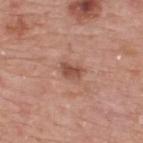No biopsy was performed on this lesion — it was imaged during a full skin examination and was not determined to be concerning. The lesion is located on the upper back. This is a white-light tile. This image is a 15 mm lesion crop taken from a total-body photograph. The patient is a male about 75 years old.